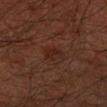Q: Is there a histopathology result?
A: total-body-photography surveillance lesion; no biopsy
Q: How large is the lesion?
A: ≈2.5 mm
Q: Where on the body is the lesion?
A: the arm
Q: Who is the patient?
A: male, aged around 60
Q: What did automated image analysis measure?
A: about 5 CIELAB-L* units darker than the surrounding skin; a classifier nevus-likeness of about 55/100 and a lesion-detection confidence of about 100/100
Q: How was this image acquired?
A: ~15 mm crop, total-body skin-cancer survey